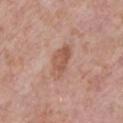follow-up = total-body-photography surveillance lesion; no biopsy | patient = male, aged 78–82 | illumination = white-light | site = the chest | image = ~15 mm tile from a whole-body skin photo.